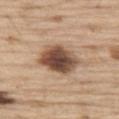size = ~5 mm (longest diameter); acquisition = total-body-photography crop, ~15 mm field of view; subject = male, approximately 70 years of age; lighting = white-light; body site = the left thigh.